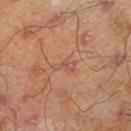Clinical impression: The lesion was photographed on a routine skin check and not biopsied; there is no pathology result. Clinical summary: A 15 mm crop from a total-body photograph taken for skin-cancer surveillance. The tile uses cross-polarized illumination. Longest diameter approximately 2.5 mm. On the leg. A male patient about 45 years old.